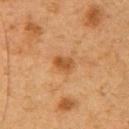Findings:
* body site — the right upper arm
* imaging modality — 15 mm crop, total-body photography
* subject — male, in their mid-60s